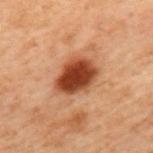image = ~15 mm crop, total-body skin-cancer survey
site = the upper back
patient = male, roughly 70 years of age
tile lighting = cross-polarized illumination
automated lesion analysis = a lesion area of about 14 mm² and two-axis asymmetry of about 0.2; a mean CIELAB color near L≈34 a*≈22 b*≈29; border irregularity of about 2 on a 0–10 scale; a nevus-likeness score of about 100/100 and a detector confidence of about 100 out of 100 that the crop contains a lesion
lesion diameter = ~5 mm (longest diameter)